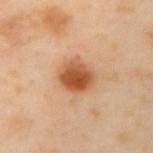biopsy status: imaged on a skin check; not biopsied | anatomic site: the mid back | image: 15 mm crop, total-body photography | subject: female, aged around 55 | lighting: cross-polarized illumination.